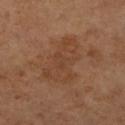Case summary:
- follow-up: no biopsy performed (imaged during a skin exam)
- tile lighting: cross-polarized
- lesion diameter: ~6 mm (longest diameter)
- site: the right lower leg
- subject: female, aged approximately 65
- imaging modality: ~15 mm tile from a whole-body skin photo
- TBP lesion metrics: an eccentricity of roughly 0.8; a mean CIELAB color near L≈40 a*≈19 b*≈30, roughly 6 lightness units darker than nearby skin, and a lesion-to-skin contrast of about 5 (normalized; higher = more distinct); a border-irregularity index near 4.5/10 and a color-variation rating of about 2.5/10